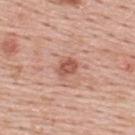Impression: Captured during whole-body skin photography for melanoma surveillance; the lesion was not biopsied. Background: Cropped from a whole-body photographic skin survey; the tile spans about 15 mm. The lesion's longest dimension is about 2.5 mm. An algorithmic analysis of the crop reported an average lesion color of about L≈55 a*≈26 b*≈29 (CIELAB) and a normalized lesion–skin contrast near 7.5. The lesion is located on the upper back. A male subject approximately 55 years of age.